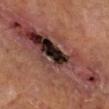Image and clinical context:
The tile uses cross-polarized illumination. The recorded lesion diameter is about 15 mm. The subject is a male aged around 65. This image is a 15 mm lesion crop taken from a total-body photograph. On the left leg. The lesion-visualizer software estimated an average lesion color of about L≈38 a*≈22 b*≈21 (CIELAB), a lesion–skin lightness drop of about 11, and a normalized border contrast of about 11. The software also gave a border-irregularity index near 6.5/10, a color-variation rating of about 10/10, and a peripheral color-asymmetry measure near 5.5.